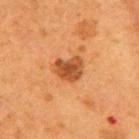Impression:
Recorded during total-body skin imaging; not selected for excision or biopsy.
Acquisition and patient details:
The subject is a female about 50 years old. A 15 mm close-up extracted from a 3D total-body photography capture. The total-body-photography lesion software estimated a footprint of about 7.5 mm² and a shape eccentricity near 0.55. The analysis additionally found border irregularity of about 2.5 on a 0–10 scale, internal color variation of about 3.5 on a 0–10 scale, and radial color variation of about 1. And it measured an automated nevus-likeness rating near 90 out of 100 and a detector confidence of about 100 out of 100 that the crop contains a lesion. The lesion is on the mid back.